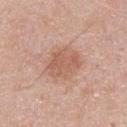workup — no biopsy performed (imaged during a skin exam); lighting — white-light illumination; diameter — ~4 mm (longest diameter); patient — male, about 45 years old; image — 15 mm crop, total-body photography; body site — the upper back.